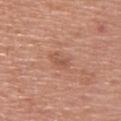The lesion was photographed on a routine skin check and not biopsied; there is no pathology result. A roughly 15 mm field-of-view crop from a total-body skin photograph. A female subject, aged 48 to 52. The tile uses white-light illumination. The lesion is on the upper back.